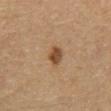Q: Was a biopsy performed?
A: total-body-photography surveillance lesion; no biopsy
Q: What is the anatomic site?
A: the chest
Q: How was this image acquired?
A: ~15 mm crop, total-body skin-cancer survey
Q: Patient demographics?
A: male, aged 63–67
Q: How large is the lesion?
A: ~2.5 mm (longest diameter)